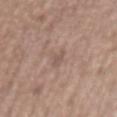The lesion was tiled from a total-body skin photograph and was not biopsied. This is a white-light tile. The lesion's longest dimension is about 2.5 mm. On the back. A 15 mm close-up extracted from a 3D total-body photography capture. A female patient, in their mid- to late 60s.